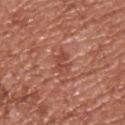Impression: The lesion was tiled from a total-body skin photograph and was not biopsied. Background: Cropped from a whole-body photographic skin survey; the tile spans about 15 mm. The subject is a male aged 53–57. Captured under white-light illumination. Approximately 2.5 mm at its widest. The lesion is located on the upper back.